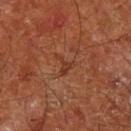Assessment:
The lesion was photographed on a routine skin check and not biopsied; there is no pathology result.
Image and clinical context:
Located on the left lower leg. Automated tile analysis of the lesion measured an outline eccentricity of about 0.7 (0 = round, 1 = elongated) and a shape-asymmetry score of about 0.75 (0 = symmetric). The software also gave a lesion color around L≈35 a*≈24 b*≈31 in CIELAB, roughly 6 lightness units darker than nearby skin, and a normalized lesion–skin contrast near 6. And it measured a border-irregularity index near 7.5/10 and peripheral color asymmetry of about 0. Cropped from a total-body skin-imaging series; the visible field is about 15 mm. The tile uses cross-polarized illumination. The patient is a male aged approximately 65.